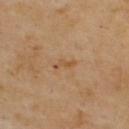biopsy status: catalogued during a skin exam; not biopsied | patient: male, approximately 55 years of age | location: the upper back | lesion diameter: ~3 mm (longest diameter) | image source: 15 mm crop, total-body photography | illumination: cross-polarized illumination.